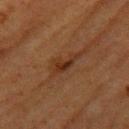• location: the left upper arm
• subject: female, aged 53 to 57
• image source: total-body-photography crop, ~15 mm field of view
• size: ≈4.5 mm